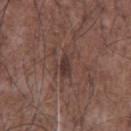<lesion>
  <biopsy_status>not biopsied; imaged during a skin examination</biopsy_status>
  <patient>
    <sex>male</sex>
    <age_approx>70</age_approx>
  </patient>
  <lesion_size>
    <long_diameter_mm_approx>3.0</long_diameter_mm_approx>
  </lesion_size>
  <image>
    <source>total-body photography crop</source>
    <field_of_view_mm>15</field_of_view_mm>
  </image>
  <site>chest</site>
</lesion>